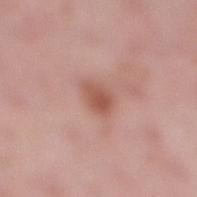Notes:
– workup: total-body-photography surveillance lesion; no biopsy
– body site: the right lower leg
– subject: female, about 50 years old
– acquisition: ~15 mm tile from a whole-body skin photo
– illumination: white-light
– diameter: ~3 mm (longest diameter)